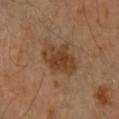Captured during whole-body skin photography for melanoma surveillance; the lesion was not biopsied. The subject is a female roughly 60 years of age. The lesion is on the arm. A region of skin cropped from a whole-body photographic capture, roughly 15 mm wide. Measured at roughly 5 mm in maximum diameter. The total-body-photography lesion software estimated an outline eccentricity of about 0.75 (0 = round, 1 = elongated) and a symmetry-axis asymmetry near 0.15. The analysis additionally found a classifier nevus-likeness of about 65/100 and lesion-presence confidence of about 100/100.